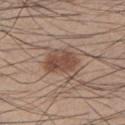This lesion was catalogued during total-body skin photography and was not selected for biopsy. The lesion is on the left lower leg. The subject is a male about 55 years old. This image is a 15 mm lesion crop taken from a total-body photograph. Imaged with white-light lighting. An algorithmic analysis of the crop reported a border-irregularity index near 3/10, a within-lesion color-variation index near 4/10, and a peripheral color-asymmetry measure near 1.5. The software also gave lesion-presence confidence of about 100/100.